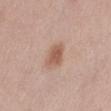| field | value |
|---|---|
| notes | imaged on a skin check; not biopsied |
| site | the left thigh |
| image | 15 mm crop, total-body photography |
| subject | female, about 40 years old |